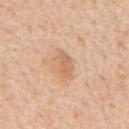follow-up — no biopsy performed (imaged during a skin exam); subject — male, aged around 70; body site — the mid back; size — ≈3.5 mm; imaging modality — ~15 mm tile from a whole-body skin photo; tile lighting — white-light.